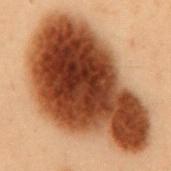{
  "biopsy_status": "not biopsied; imaged during a skin examination"
}